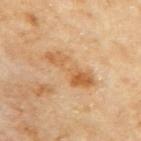Captured during whole-body skin photography for melanoma surveillance; the lesion was not biopsied.
A 15 mm close-up extracted from a 3D total-body photography capture.
A female patient aged 58–62.
Located on the right upper arm.